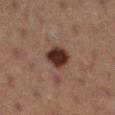| key | value |
|---|---|
| biopsy status | imaged on a skin check; not biopsied |
| size | ≈3 mm |
| subject | female, aged 38–42 |
| image | ~15 mm crop, total-body skin-cancer survey |
| lighting | cross-polarized illumination |
| site | the left lower leg |
| automated metrics | a lesion color around L≈25 a*≈16 b*≈20 in CIELAB and a normalized border contrast of about 14.5; an automated nevus-likeness rating near 100 out of 100 |